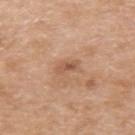workup: catalogued during a skin exam; not biopsied
anatomic site: the arm
TBP lesion metrics: an outline eccentricity of about 0.85 (0 = round, 1 = elongated) and a symmetry-axis asymmetry near 0.35; a normalized border contrast of about 6; a color-variation rating of about 1/10; a classifier nevus-likeness of about 0/100
illumination: white-light illumination
subject: male, roughly 60 years of age
acquisition: total-body-photography crop, ~15 mm field of view